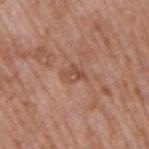Case summary:
* patient: male, aged 68–72
* body site: the arm
* image source: 15 mm crop, total-body photography